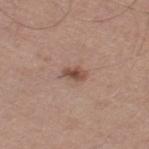<record>
  <biopsy_status>not biopsied; imaged during a skin examination</biopsy_status>
  <image>
    <source>total-body photography crop</source>
    <field_of_view_mm>15</field_of_view_mm>
  </image>
  <patient>
    <sex>male</sex>
    <age_approx>50</age_approx>
  </patient>
  <site>right thigh</site>
</record>